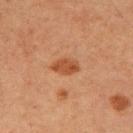| feature | finding |
|---|---|
| follow-up | catalogued during a skin exam; not biopsied |